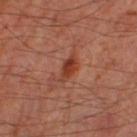This lesion was catalogued during total-body skin photography and was not selected for biopsy.
The patient is a male aged 63–67.
This is a cross-polarized tile.
From the right thigh.
A lesion tile, about 15 mm wide, cut from a 3D total-body photograph.
Longest diameter approximately 3.5 mm.